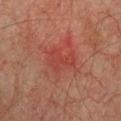biopsy_status: not biopsied; imaged during a skin examination
patient:
  sex: male
  age_approx: 75
site: chest
lesion_size:
  long_diameter_mm_approx: 3.5
lighting: cross-polarized
image:
  source: total-body photography crop
  field_of_view_mm: 15
automated_metrics:
  cielab_L: 35
  cielab_a: 28
  cielab_b: 26
  vs_skin_contrast_norm: 4.5
  border_irregularity_0_10: 7.5
  color_variation_0_10: 1.0
  peripheral_color_asymmetry: 0.5